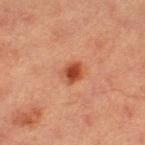Recorded during total-body skin imaging; not selected for excision or biopsy. The total-body-photography lesion software estimated a lesion area of about 5.5 mm², an outline eccentricity of about 0.5 (0 = round, 1 = elongated), and a symmetry-axis asymmetry near 0.15. The analysis additionally found border irregularity of about 1.5 on a 0–10 scale, internal color variation of about 4.5 on a 0–10 scale, and peripheral color asymmetry of about 1.5. On the left thigh. A female subject, aged 38–42. The recorded lesion diameter is about 3 mm. A 15 mm crop from a total-body photograph taken for skin-cancer surveillance.